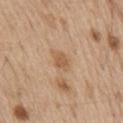Q: Was a biopsy performed?
A: no biopsy performed (imaged during a skin exam)
Q: Who is the patient?
A: male, about 70 years old
Q: What is the anatomic site?
A: the mid back
Q: What kind of image is this?
A: ~15 mm tile from a whole-body skin photo